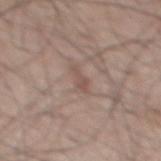Q: Is there a histopathology result?
A: total-body-photography surveillance lesion; no biopsy
Q: What are the patient's age and sex?
A: male, aged approximately 50
Q: What is the imaging modality?
A: total-body-photography crop, ~15 mm field of view
Q: What is the anatomic site?
A: the back